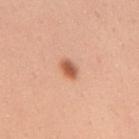No biopsy was performed on this lesion — it was imaged during a full skin examination and was not determined to be concerning.
The patient is a female aged 33 to 37.
On the upper back.
Captured under white-light illumination.
A 15 mm close-up extracted from a 3D total-body photography capture.
Longest diameter approximately 2.5 mm.
An algorithmic analysis of the crop reported an average lesion color of about L≈59 a*≈26 b*≈35 (CIELAB), about 14 CIELAB-L* units darker than the surrounding skin, and a normalized border contrast of about 8.5. The software also gave border irregularity of about 1.5 on a 0–10 scale, a color-variation rating of about 3/10, and a peripheral color-asymmetry measure near 1.